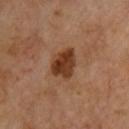workup=total-body-photography surveillance lesion; no biopsy | imaging modality=~15 mm crop, total-body skin-cancer survey | body site=the chest | diameter=about 4 mm | patient=male, aged approximately 70 | automated lesion analysis=a mean CIELAB color near L≈36 a*≈21 b*≈32, roughly 13 lightness units darker than nearby skin, and a normalized border contrast of about 10.5 | tile lighting=cross-polarized illumination.